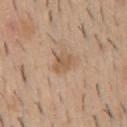biopsy_status: not biopsied; imaged during a skin examination
lighting: white-light
patient:
  sex: male
  age_approx: 40
site: front of the torso
image:
  source: total-body photography crop
  field_of_view_mm: 15